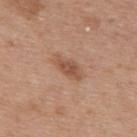Notes:
* notes: imaged on a skin check; not biopsied
* location: the upper back
* lesion diameter: ≈4 mm
* patient: female, aged 38 to 42
* imaging modality: ~15 mm tile from a whole-body skin photo
* lighting: white-light illumination